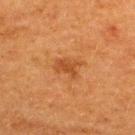{
  "biopsy_status": "not biopsied; imaged during a skin examination",
  "site": "upper back",
  "patient": {
    "sex": "female",
    "age_approx": 40
  },
  "automated_metrics": {
    "area_mm2_approx": 5.0,
    "shape_asymmetry": 0.35,
    "border_irregularity_0_10": 4.5,
    "color_variation_0_10": 1.5,
    "nevus_likeness_0_100": 15,
    "lesion_detection_confidence_0_100": 100
  },
  "lesion_size": {
    "long_diameter_mm_approx": 3.0
  },
  "lighting": "cross-polarized",
  "image": {
    "source": "total-body photography crop",
    "field_of_view_mm": 15
  }
}